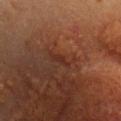Part of a total-body skin-imaging series; this lesion was reviewed on a skin check and was not flagged for biopsy. The lesion is on the chest. A lesion tile, about 15 mm wide, cut from a 3D total-body photograph. A female subject, aged approximately 40.